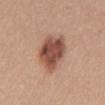notes = imaged on a skin check; not biopsied
subject = female, roughly 40 years of age
body site = the mid back
image source = ~15 mm crop, total-body skin-cancer survey